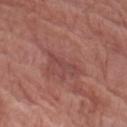  patient:
    sex: female
    age_approx: 80
  lesion_size:
    long_diameter_mm_approx: 3.5
  image:
    source: total-body photography crop
    field_of_view_mm: 15
  automated_metrics:
    area_mm2_approx: 3.0
    eccentricity: 0.95
    shape_asymmetry: 0.5
    cielab_L: 45
    cielab_a: 25
    cielab_b: 23
    vs_skin_darker_L: 6.0
    vs_skin_contrast_norm: 5.0
    border_irregularity_0_10: 7.0
    color_variation_0_10: 0.0
    peripheral_color_asymmetry: 0.0
    nevus_likeness_0_100: 0
    lesion_detection_confidence_0_100: 75
  site: left forearm